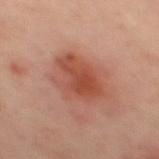Findings:
– biopsy status: no biopsy performed (imaged during a skin exam)
– subject: male, aged around 50
– site: the mid back
– lesion size: ~5.5 mm (longest diameter)
– imaging modality: ~15 mm crop, total-body skin-cancer survey
– tile lighting: cross-polarized illumination